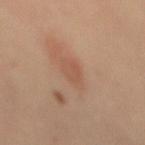Q: Was a biopsy performed?
A: catalogued during a skin exam; not biopsied
Q: Automated lesion metrics?
A: a lesion area of about 5 mm², an outline eccentricity of about 0.85 (0 = round, 1 = elongated), and a symmetry-axis asymmetry near 0.25; internal color variation of about 1.5 on a 0–10 scale and peripheral color asymmetry of about 0.5; a nevus-likeness score of about 5/100
Q: What is the anatomic site?
A: the mid back
Q: Who is the patient?
A: female, about 45 years old
Q: What is the imaging modality?
A: ~15 mm crop, total-body skin-cancer survey